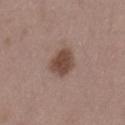| key | value |
|---|---|
| biopsy status | catalogued during a skin exam; not biopsied |
| image | total-body-photography crop, ~15 mm field of view |
| location | the lower back |
| patient | male, about 50 years old |